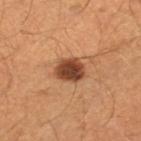Q: Was a biopsy performed?
A: total-body-photography surveillance lesion; no biopsy
Q: What is the anatomic site?
A: the right lower leg
Q: How large is the lesion?
A: ≈3.5 mm
Q: What are the patient's age and sex?
A: male, aged around 40
Q: What is the imaging modality?
A: ~15 mm tile from a whole-body skin photo
Q: Illumination type?
A: cross-polarized illumination